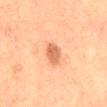| feature | finding |
|---|---|
| notes | total-body-photography surveillance lesion; no biopsy |
| subject | female, roughly 70 years of age |
| image | 15 mm crop, total-body photography |
| lesion size | about 3.5 mm |
| illumination | cross-polarized |
| body site | the mid back |